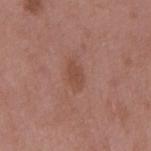Part of a total-body skin-imaging series; this lesion was reviewed on a skin check and was not flagged for biopsy. The lesion is located on the mid back. This image is a 15 mm lesion crop taken from a total-body photograph. Automated image analysis of the tile measured a footprint of about 3.5 mm², an outline eccentricity of about 0.85 (0 = round, 1 = elongated), and a shape-asymmetry score of about 0.25 (0 = symmetric). The software also gave a lesion color around L≈47 a*≈23 b*≈28 in CIELAB, roughly 7 lightness units darker than nearby skin, and a normalized lesion–skin contrast near 6. The analysis additionally found a border-irregularity rating of about 3/10 and internal color variation of about 1.5 on a 0–10 scale. The software also gave a classifier nevus-likeness of about 5/100 and lesion-presence confidence of about 100/100. Imaged with white-light lighting. A male patient, about 50 years old.